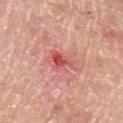follow-up: total-body-photography surveillance lesion; no biopsy
image source: 15 mm crop, total-body photography
patient: male, approximately 80 years of age
tile lighting: white-light
lesion size: ~3.5 mm (longest diameter)
location: the left upper arm